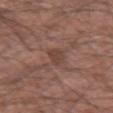{"biopsy_status": "not biopsied; imaged during a skin examination", "site": "arm", "patient": {"sex": "male", "age_approx": 50}, "automated_metrics": {"area_mm2_approx": 4.0, "eccentricity": 0.85, "border_irregularity_0_10": 2.5, "color_variation_0_10": 1.5}, "image": {"source": "total-body photography crop", "field_of_view_mm": 15}, "lesion_size": {"long_diameter_mm_approx": 3.0}}